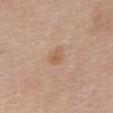Captured during whole-body skin photography for melanoma surveillance; the lesion was not biopsied. Longest diameter approximately 2.5 mm. A roughly 15 mm field-of-view crop from a total-body skin photograph. From the mid back. A female patient in their mid- to late 60s.